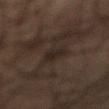Clinical impression:
Part of a total-body skin-imaging series; this lesion was reviewed on a skin check and was not flagged for biopsy.
Image and clinical context:
From the abdomen. Measured at roughly 2.5 mm in maximum diameter. Captured under cross-polarized illumination. A close-up tile cropped from a whole-body skin photograph, about 15 mm across. The total-body-photography lesion software estimated a lesion color around L≈20 a*≈9 b*≈15 in CIELAB, roughly 5 lightness units darker than nearby skin, and a normalized border contrast of about 7. The analysis additionally found a border-irregularity index near 3/10, a within-lesion color-variation index near 2/10, and peripheral color asymmetry of about 1. And it measured an automated nevus-likeness rating near 5 out of 100 and lesion-presence confidence of about 65/100. A male patient, in their mid- to late 50s.